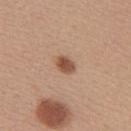biopsy status: catalogued during a skin exam; not biopsied
lesion size: ~2.5 mm (longest diameter)
patient: female, aged around 40
site: the back
illumination: white-light
image source: ~15 mm crop, total-body skin-cancer survey